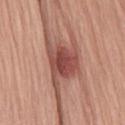<record>
<biopsy_status>not biopsied; imaged during a skin examination</biopsy_status>
<site>lower back</site>
<patient>
  <sex>male</sex>
  <age_approx>55</age_approx>
</patient>
<lesion_size>
  <long_diameter_mm_approx>8.5</long_diameter_mm_approx>
</lesion_size>
<image>
  <source>total-body photography crop</source>
  <field_of_view_mm>15</field_of_view_mm>
</image>
</record>